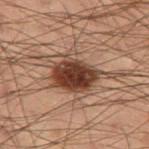Part of a total-body skin-imaging series; this lesion was reviewed on a skin check and was not flagged for biopsy.
The recorded lesion diameter is about 7.5 mm.
A 15 mm crop from a total-body photograph taken for skin-cancer surveillance.
Captured under cross-polarized illumination.
The patient is a male in their mid- to late 50s.
The lesion is located on the left thigh.